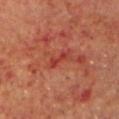Q: Is there a histopathology result?
A: no biopsy performed (imaged during a skin exam)
Q: Lesion location?
A: the head or neck
Q: Who is the patient?
A: male, aged approximately 70
Q: How was this image acquired?
A: 15 mm crop, total-body photography